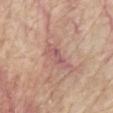| field | value |
|---|---|
| biopsy status | total-body-photography surveillance lesion; no biopsy |
| site | the chest |
| subject | male, approximately 65 years of age |
| image source | total-body-photography crop, ~15 mm field of view |